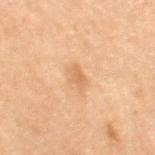| field | value |
|---|---|
| notes | total-body-photography surveillance lesion; no biopsy |
| subject | female, roughly 55 years of age |
| site | the right thigh |
| lesion diameter | about 3 mm |
| illumination | cross-polarized |
| image source | 15 mm crop, total-body photography |
| automated metrics | a lesion–skin lightness drop of about 7 and a normalized lesion–skin contrast near 5.5 |